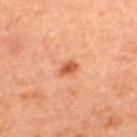Q: How was this image acquired?
A: ~15 mm tile from a whole-body skin photo
Q: What are the patient's age and sex?
A: female, in their mid- to late 40s
Q: Lesion location?
A: the upper back